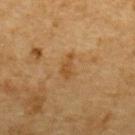Q: Was this lesion biopsied?
A: no biopsy performed (imaged during a skin exam)
Q: What lighting was used for the tile?
A: cross-polarized illumination
Q: Patient demographics?
A: male, in their mid-80s or older
Q: What is the lesion's diameter?
A: ~2.5 mm (longest diameter)
Q: How was this image acquired?
A: 15 mm crop, total-body photography
Q: What is the anatomic site?
A: the upper back